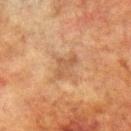Assessment:
Recorded during total-body skin imaging; not selected for excision or biopsy.
Context:
A roughly 15 mm field-of-view crop from a total-body skin photograph. This is a cross-polarized tile. The subject is a male approximately 75 years of age. On the right upper arm. Automated image analysis of the tile measured a nevus-likeness score of about 0/100 and a detector confidence of about 100 out of 100 that the crop contains a lesion.